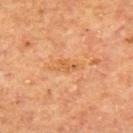The lesion was photographed on a routine skin check and not biopsied; there is no pathology result. A male patient aged 63 to 67. Automated image analysis of the tile measured an area of roughly 5 mm² and a shape-asymmetry score of about 0.25 (0 = symmetric). It also reported an average lesion color of about L≈55 a*≈23 b*≈39 (CIELAB) and a lesion–skin lightness drop of about 6. And it measured a border-irregularity index near 3.5/10 and a within-lesion color-variation index near 2.5/10. The recorded lesion diameter is about 4 mm. A region of skin cropped from a whole-body photographic capture, roughly 15 mm wide. On the upper back.